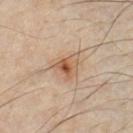The lesion was tiled from a total-body skin photograph and was not biopsied.
About 3.5 mm across.
A male subject, in their mid-30s.
A region of skin cropped from a whole-body photographic capture, roughly 15 mm wide.
Imaged with cross-polarized lighting.
The lesion is on the chest.